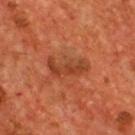Impression:
Part of a total-body skin-imaging series; this lesion was reviewed on a skin check and was not flagged for biopsy.
Acquisition and patient details:
The lesion is on the chest. Captured under cross-polarized illumination. About 4.5 mm across. A male subject, aged 53 to 57. A roughly 15 mm field-of-view crop from a total-body skin photograph.